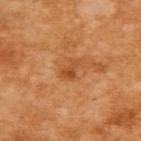Case summary:
• workup: total-body-photography surveillance lesion; no biopsy
• imaging modality: ~15 mm tile from a whole-body skin photo
• lesion size: ≈2.5 mm
• subject: female, aged 53–57
• illumination: cross-polarized
• body site: the upper back